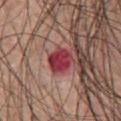biopsy status = catalogued during a skin exam; not biopsied
lesion diameter = ~3 mm (longest diameter)
automated metrics = a lesion area of about 7 mm² and a shape eccentricity near 0.55; a border-irregularity rating of about 1.5/10, internal color variation of about 3.5 on a 0–10 scale, and a peripheral color-asymmetry measure near 1; a nevus-likeness score of about 0/100 and lesion-presence confidence of about 100/100
subject = male, aged around 65
lighting = white-light illumination
anatomic site = the front of the torso
acquisition = 15 mm crop, total-body photography